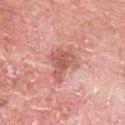{"biopsy_status": "not biopsied; imaged during a skin examination", "image": {"source": "total-body photography crop", "field_of_view_mm": 15}, "patient": {"sex": "male", "age_approx": 80}, "site": "left upper arm"}